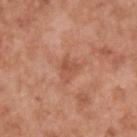Case summary:
• workup · total-body-photography surveillance lesion; no biopsy
• image source · ~15 mm crop, total-body skin-cancer survey
• body site · the upper back
• subject · male, aged approximately 65